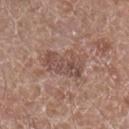The lesion was tiled from a total-body skin photograph and was not biopsied.
The subject is a male approximately 60 years of age.
A 15 mm close-up extracted from a 3D total-body photography capture.
Located on the left forearm.